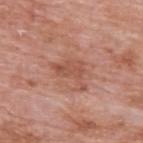This lesion was catalogued during total-body skin photography and was not selected for biopsy. A male subject, approximately 60 years of age. Longest diameter approximately 5 mm. This image is a 15 mm lesion crop taken from a total-body photograph. The lesion is located on the upper back. The total-body-photography lesion software estimated a mean CIELAB color near L≈53 a*≈25 b*≈30, roughly 8 lightness units darker than nearby skin, and a normalized border contrast of about 6. The tile uses white-light illumination.